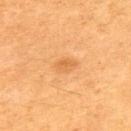| feature | finding |
|---|---|
| anatomic site | the upper back |
| imaging modality | 15 mm crop, total-body photography |
| image-analysis metrics | a shape eccentricity near 0.75 and two-axis asymmetry of about 0.25; a border-irregularity rating of about 2.5/10, a within-lesion color-variation index near 2/10, and peripheral color asymmetry of about 0.5; a nevus-likeness score of about 20/100 |
| size | ~2.5 mm (longest diameter) |
| patient | male, aged 58 to 62 |